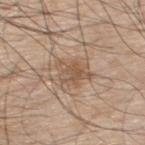Recorded during total-body skin imaging; not selected for excision or biopsy. A male patient roughly 70 years of age. A 15 mm crop from a total-body photograph taken for skin-cancer surveillance. The lesion is located on the back.